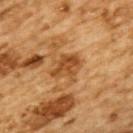Clinical impression: This lesion was catalogued during total-body skin photography and was not selected for biopsy. Image and clinical context: The subject is a male in their mid-80s or older. A 15 mm close-up tile from a total-body photography series done for melanoma screening. This is a cross-polarized tile. From the back. Measured at roughly 3.5 mm in maximum diameter.